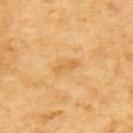Recorded during total-body skin imaging; not selected for excision or biopsy.
The lesion is on the upper back.
Longest diameter approximately 3 mm.
The subject is a male approximately 85 years of age or older.
Cropped from a total-body skin-imaging series; the visible field is about 15 mm.
The tile uses cross-polarized illumination.
Automated tile analysis of the lesion measured an area of roughly 2.5 mm² and two-axis asymmetry of about 0.4. The software also gave a lesion color around L≈55 a*≈18 b*≈42 in CIELAB, roughly 6 lightness units darker than nearby skin, and a lesion-to-skin contrast of about 5 (normalized; higher = more distinct). The software also gave a border-irregularity index near 4/10 and radial color variation of about 0. And it measured a nevus-likeness score of about 0/100 and a detector confidence of about 100 out of 100 that the crop contains a lesion.